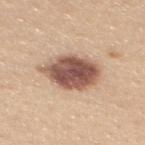No biopsy was performed on this lesion — it was imaged during a full skin examination and was not determined to be concerning. This image is a 15 mm lesion crop taken from a total-body photograph. The tile uses white-light illumination. On the upper back. Automated image analysis of the tile measured an eccentricity of roughly 0.7 and two-axis asymmetry of about 0.15. The software also gave an automated nevus-likeness rating near 95 out of 100. A female subject in their mid-20s. Longest diameter approximately 6 mm.